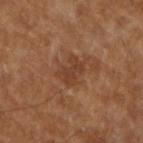Recorded during total-body skin imaging; not selected for excision or biopsy.
Located on the arm.
A 15 mm close-up extracted from a 3D total-body photography capture.
The subject is a male about 60 years old.